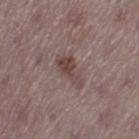Imaged during a routine full-body skin examination; the lesion was not biopsied and no histopathology is available.